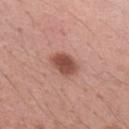Recorded during total-body skin imaging; not selected for excision or biopsy. On the right upper arm. Cropped from a total-body skin-imaging series; the visible field is about 15 mm. The total-body-photography lesion software estimated a lesion area of about 7 mm² and a shape-asymmetry score of about 0.2 (0 = symmetric). It also reported a mean CIELAB color near L≈50 a*≈24 b*≈27. A female patient, aged approximately 35. Measured at roughly 3.5 mm in maximum diameter. This is a white-light tile.